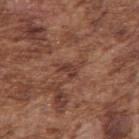notes = no biopsy performed (imaged during a skin exam).